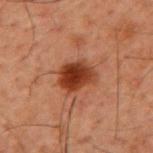This lesion was catalogued during total-body skin photography and was not selected for biopsy.
A roughly 15 mm field-of-view crop from a total-body skin photograph.
A male subject, aged 58–62.
This is a cross-polarized tile.
About 4 mm across.
Located on the back.